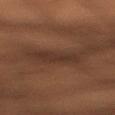Clinical impression: Imaged during a routine full-body skin examination; the lesion was not biopsied and no histopathology is available. Image and clinical context: A 15 mm crop from a total-body photograph taken for skin-cancer surveillance. The subject is a male aged 48–52. The lesion is located on the right lower leg. Captured under cross-polarized illumination. The total-body-photography lesion software estimated a lesion area of about 5.5 mm² and two-axis asymmetry of about 0.35. It also reported a lesion color around L≈24 a*≈14 b*≈20 in CIELAB, a lesion–skin lightness drop of about 4, and a lesion-to-skin contrast of about 5.5 (normalized; higher = more distinct). It also reported an automated nevus-likeness rating near 0 out of 100. About 3 mm across.